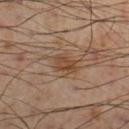  biopsy_status: not biopsied; imaged during a skin examination
  site: leg
  image:
    source: total-body photography crop
    field_of_view_mm: 15
  patient:
    sex: male
    age_approx: 55